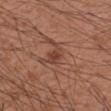Case summary:
* biopsy status — imaged on a skin check; not biopsied
* site — the right forearm
* image — total-body-photography crop, ~15 mm field of view
* patient — male, aged approximately 30